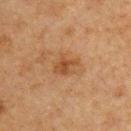Assessment:
This lesion was catalogued during total-body skin photography and was not selected for biopsy.
Context:
Imaged with cross-polarized lighting. Cropped from a total-body skin-imaging series; the visible field is about 15 mm. About 3.5 mm across. The lesion is located on the chest. A male patient, aged around 75. An algorithmic analysis of the crop reported a border-irregularity rating of about 3.5/10, a within-lesion color-variation index near 3/10, and radial color variation of about 1. And it measured an automated nevus-likeness rating near 15 out of 100 and a detector confidence of about 100 out of 100 that the crop contains a lesion.